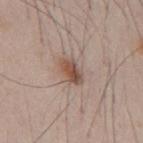follow-up=imaged on a skin check; not biopsied | tile lighting=white-light | acquisition=total-body-photography crop, ~15 mm field of view | lesion size=about 3.5 mm | body site=the back | subject=male, in their mid- to late 30s.